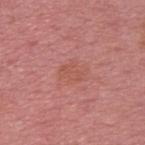This lesion was catalogued during total-body skin photography and was not selected for biopsy. A male patient, about 55 years old. A roughly 15 mm field-of-view crop from a total-body skin photograph. On the upper back. About 3 mm across. An algorithmic analysis of the crop reported an area of roughly 5 mm² and an outline eccentricity of about 0.65 (0 = round, 1 = elongated). The analysis additionally found a detector confidence of about 100 out of 100 that the crop contains a lesion. Captured under white-light illumination.